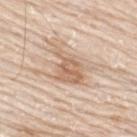The lesion is on the upper back.
A male patient approximately 75 years of age.
A close-up tile cropped from a whole-body skin photograph, about 15 mm across.
The lesion-visualizer software estimated a lesion area of about 10 mm², an outline eccentricity of about 0.75 (0 = round, 1 = elongated), and a shape-asymmetry score of about 0.4 (0 = symmetric). And it measured a lesion color around L≈64 a*≈18 b*≈31 in CIELAB and roughly 10 lightness units darker than nearby skin. And it measured a border-irregularity index near 5/10, a color-variation rating of about 4/10, and radial color variation of about 1.5.